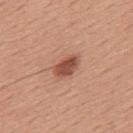workup: catalogued during a skin exam; not biopsied | image: ~15 mm tile from a whole-body skin photo | subject: male, in their 50s | image-analysis metrics: an area of roughly 6 mm², an outline eccentricity of about 0.8 (0 = round, 1 = elongated), and a shape-asymmetry score of about 0.2 (0 = symmetric); a mean CIELAB color near L≈51 a*≈25 b*≈30, a lesion–skin lightness drop of about 13, and a lesion-to-skin contrast of about 9 (normalized; higher = more distinct); a nevus-likeness score of about 95/100 and lesion-presence confidence of about 100/100 | illumination: white-light | diameter: ~3.5 mm (longest diameter) | site: the back.